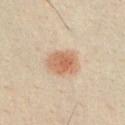| feature | finding |
|---|---|
| lighting | cross-polarized |
| body site | the chest |
| size | ≈3.5 mm |
| subject | male, aged 48 to 52 |
| imaging modality | total-body-photography crop, ~15 mm field of view |